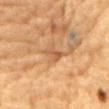Assessment:
This lesion was catalogued during total-body skin photography and was not selected for biopsy.
Background:
Captured under cross-polarized illumination. A lesion tile, about 15 mm wide, cut from a 3D total-body photograph. The lesion's longest dimension is about 2.5 mm. From the chest. A male subject aged 83 or older.